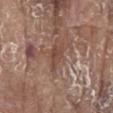Notes:
– biopsy status: catalogued during a skin exam; not biopsied
– subject: male, approximately 80 years of age
– illumination: white-light illumination
– anatomic site: the chest
– imaging modality: 15 mm crop, total-body photography
– size: ≈3.5 mm